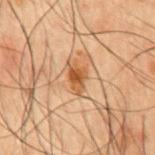  biopsy_status: not biopsied; imaged during a skin examination
  site: abdomen
  patient:
    sex: male
    age_approx: 50
  image:
    source: total-body photography crop
    field_of_view_mm: 15